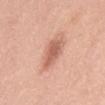The lesion was tiled from a total-body skin photograph and was not biopsied. The subject is a female aged 38–42. Measured at roughly 4 mm in maximum diameter. A lesion tile, about 15 mm wide, cut from a 3D total-body photograph. Automated image analysis of the tile measured a classifier nevus-likeness of about 75/100 and lesion-presence confidence of about 100/100. From the mid back.